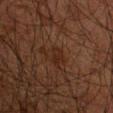Clinical impression:
No biopsy was performed on this lesion — it was imaged during a full skin examination and was not determined to be concerning.